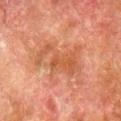Captured during whole-body skin photography for melanoma surveillance; the lesion was not biopsied. A male patient approximately 80 years of age. Approximately 6.5 mm at its widest. Automated tile analysis of the lesion measured an area of roughly 15 mm² and a shape eccentricity near 0.85. The software also gave an average lesion color of about L≈43 a*≈23 b*≈31 (CIELAB), roughly 6 lightness units darker than nearby skin, and a normalized lesion–skin contrast near 6. The analysis additionally found a within-lesion color-variation index near 2.5/10 and peripheral color asymmetry of about 1. A 15 mm close-up tile from a total-body photography series done for melanoma screening. From the right lower leg. This is a cross-polarized tile.